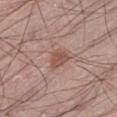Captured during whole-body skin photography for melanoma surveillance; the lesion was not biopsied.
Cropped from a total-body skin-imaging series; the visible field is about 15 mm.
The tile uses white-light illumination.
The lesion is located on the left lower leg.
A male patient, aged approximately 50.
Longest diameter approximately 2.5 mm.